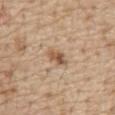Captured during whole-body skin photography for melanoma surveillance; the lesion was not biopsied. Longest diameter approximately 3 mm. Automated image analysis of the tile measured a lesion color around L≈55 a*≈18 b*≈32 in CIELAB, about 12 CIELAB-L* units darker than the surrounding skin, and a normalized border contrast of about 8. And it measured a border-irregularity index near 2.5/10, a within-lesion color-variation index near 6/10, and radial color variation of about 2. And it measured an automated nevus-likeness rating near 80 out of 100 and a lesion-detection confidence of about 100/100. Cropped from a whole-body photographic skin survey; the tile spans about 15 mm. Captured under white-light illumination. The lesion is on the abdomen. The patient is a male aged 68 to 72.